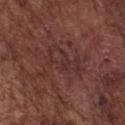Clinical impression:
Part of a total-body skin-imaging series; this lesion was reviewed on a skin check and was not flagged for biopsy.
Background:
This is a white-light tile. A male subject aged approximately 75. A 15 mm close-up tile from a total-body photography series done for melanoma screening. From the chest.